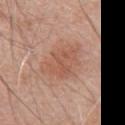The lesion was tiled from a total-body skin photograph and was not biopsied.
This is a white-light tile.
On the chest.
A 15 mm close-up extracted from a 3D total-body photography capture.
A male subject, aged 68–72.